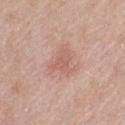Part of a total-body skin-imaging series; this lesion was reviewed on a skin check and was not flagged for biopsy.
The lesion is on the left thigh.
The subject is a female in their 60s.
The tile uses white-light illumination.
A lesion tile, about 15 mm wide, cut from a 3D total-body photograph.
An algorithmic analysis of the crop reported a footprint of about 8.5 mm², an outline eccentricity of about 0.3 (0 = round, 1 = elongated), and a symmetry-axis asymmetry near 0.55. And it measured a nevus-likeness score of about 10/100 and lesion-presence confidence of about 100/100.
Measured at roughly 3.5 mm in maximum diameter.